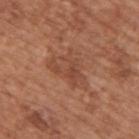Recorded during total-body skin imaging; not selected for excision or biopsy. On the upper back. A male patient, aged around 65. A 15 mm close-up extracted from a 3D total-body photography capture. This is a white-light tile. The recorded lesion diameter is about 5.5 mm.